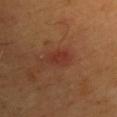Assessment: The lesion was photographed on a routine skin check and not biopsied; there is no pathology result. Clinical summary: The tile uses cross-polarized illumination. From the front of the torso. A male patient, aged approximately 65. Measured at roughly 3.5 mm in maximum diameter. A 15 mm crop from a total-body photograph taken for skin-cancer surveillance.